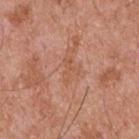This lesion was catalogued during total-body skin photography and was not selected for biopsy. The lesion is on the upper back. A male subject, aged around 55. Cropped from a whole-body photographic skin survey; the tile spans about 15 mm.